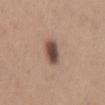Clinical impression: Imaged during a routine full-body skin examination; the lesion was not biopsied and no histopathology is available. Clinical summary: This is a white-light tile. A region of skin cropped from a whole-body photographic capture, roughly 15 mm wide. A male patient, roughly 60 years of age. The lesion is on the mid back. Longest diameter approximately 3.5 mm.